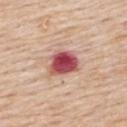| feature | finding |
|---|---|
| workup | total-body-photography surveillance lesion; no biopsy |
| body site | the back |
| acquisition | ~15 mm tile from a whole-body skin photo |
| patient | female, aged 68 to 72 |